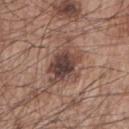The lesion was tiled from a total-body skin photograph and was not biopsied. The lesion is on the right upper arm. The patient is a male in their mid-60s. The total-body-photography lesion software estimated an average lesion color of about L≈43 a*≈18 b*≈22 (CIELAB), about 12 CIELAB-L* units darker than the surrounding skin, and a lesion-to-skin contrast of about 9.5 (normalized; higher = more distinct). And it measured an automated nevus-likeness rating near 45 out of 100 and a lesion-detection confidence of about 100/100. A 15 mm crop from a total-body photograph taken for skin-cancer surveillance. Longest diameter approximately 6 mm.